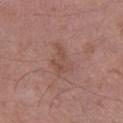Q: Is there a histopathology result?
A: imaged on a skin check; not biopsied
Q: Where on the body is the lesion?
A: the left lower leg
Q: How was this image acquired?
A: 15 mm crop, total-body photography
Q: Who is the patient?
A: male, aged around 50